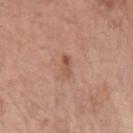Clinical impression:
Recorded during total-body skin imaging; not selected for excision or biopsy.
Background:
A 15 mm crop from a total-body photograph taken for skin-cancer surveillance. The subject is a female about 70 years old. On the left forearm. Automated tile analysis of the lesion measured an area of roughly 3 mm² and an outline eccentricity of about 0.9 (0 = round, 1 = elongated). It also reported radial color variation of about 0. Imaged with white-light lighting.